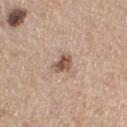Recorded during total-body skin imaging; not selected for excision or biopsy. The tile uses white-light illumination. The subject is a female roughly 70 years of age. A 15 mm close-up tile from a total-body photography series done for melanoma screening. From the right thigh. Automated tile analysis of the lesion measured a lesion–skin lightness drop of about 13 and a lesion-to-skin contrast of about 9 (normalized; higher = more distinct). It also reported a border-irregularity rating of about 3/10 and a color-variation rating of about 4.5/10. It also reported an automated nevus-likeness rating near 65 out of 100 and a detector confidence of about 100 out of 100 that the crop contains a lesion. The recorded lesion diameter is about 2.5 mm.